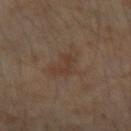Impression: Imaged during a routine full-body skin examination; the lesion was not biopsied and no histopathology is available. Acquisition and patient details: The subject is a female aged around 45. The lesion's longest dimension is about 3.5 mm. A 15 mm close-up tile from a total-body photography series done for melanoma screening. The lesion-visualizer software estimated a footprint of about 4.5 mm² and a shape-asymmetry score of about 0.5 (0 = symmetric). And it measured a classifier nevus-likeness of about 0/100 and a detector confidence of about 100 out of 100 that the crop contains a lesion. The lesion is located on the right forearm.